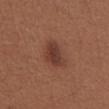<case>
<biopsy_status>not biopsied; imaged during a skin examination</biopsy_status>
<site>front of the torso</site>
<image>
  <source>total-body photography crop</source>
  <field_of_view_mm>15</field_of_view_mm>
</image>
<patient>
  <sex>female</sex>
  <age_approx>25</age_approx>
</patient>
</case>